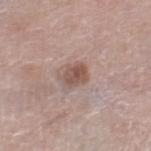follow-up=total-body-photography surveillance lesion; no biopsy
diameter=≈3 mm
subject=male, aged 78 to 82
image source=~15 mm tile from a whole-body skin photo
site=the right thigh